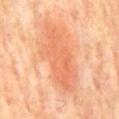biopsy_status: not biopsied; imaged during a skin examination
lighting: cross-polarized
lesion_size:
  long_diameter_mm_approx: 8.5
image:
  source: total-body photography crop
  field_of_view_mm: 15
automated_metrics:
  area_mm2_approx: 22.0
  eccentricity: 0.9
  cielab_L: 66
  cielab_a: 29
  cielab_b: 39
  vs_skin_darker_L: 9.0
  vs_skin_contrast_norm: 5.5
  lesion_detection_confidence_0_100: 100
site: back
patient:
  sex: male
  age_approx: 70